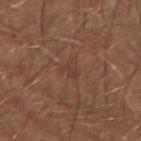Clinical impression: The lesion was photographed on a routine skin check and not biopsied; there is no pathology result. Acquisition and patient details: Imaged with white-light lighting. From the left forearm. Automated image analysis of the tile measured a shape eccentricity near 0.8 and a symmetry-axis asymmetry near 0.4. It also reported a lesion color around L≈39 a*≈18 b*≈25 in CIELAB, a lesion–skin lightness drop of about 5, and a lesion-to-skin contrast of about 4.5 (normalized; higher = more distinct). It also reported a color-variation rating of about 0.5/10 and peripheral color asymmetry of about 0. And it measured a nevus-likeness score of about 0/100. A 15 mm close-up extracted from a 3D total-body photography capture. A male subject, approximately 75 years of age.